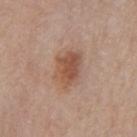Q: Was this lesion biopsied?
A: imaged on a skin check; not biopsied
Q: What are the patient's age and sex?
A: male, aged approximately 80
Q: Lesion location?
A: the chest
Q: Lesion size?
A: ≈4.5 mm
Q: Automated lesion metrics?
A: a lesion area of about 10 mm², an eccentricity of roughly 0.75, and two-axis asymmetry of about 0.25; a border-irregularity index near 3/10 and a peripheral color-asymmetry measure near 1
Q: What kind of image is this?
A: 15 mm crop, total-body photography
Q: Illumination type?
A: white-light